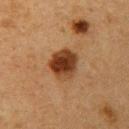The lesion was photographed on a routine skin check and not biopsied; there is no pathology result.
Cropped from a total-body skin-imaging series; the visible field is about 15 mm.
About 4 mm across.
The lesion is on the left upper arm.
A female patient, aged around 40.